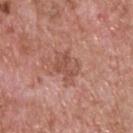Assessment:
Part of a total-body skin-imaging series; this lesion was reviewed on a skin check and was not flagged for biopsy.
Clinical summary:
The lesion's longest dimension is about 3.5 mm. A 15 mm crop from a total-body photograph taken for skin-cancer surveillance. The subject is a male aged around 60. The lesion is located on the upper back. Automated image analysis of the tile measured a lesion color around L≈52 a*≈23 b*≈28 in CIELAB, roughly 8 lightness units darker than nearby skin, and a normalized border contrast of about 5.5. And it measured a border-irregularity rating of about 4/10, a color-variation rating of about 3/10, and peripheral color asymmetry of about 1. The tile uses white-light illumination.